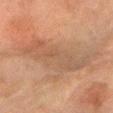  lighting: cross-polarized
  patient:
    sex: female
    age_approx: 60
  site: arm
  lesion_size:
    long_diameter_mm_approx: 7.5
  image:
    source: total-body photography crop
    field_of_view_mm: 15